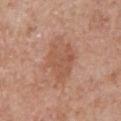biopsy status=total-body-photography surveillance lesion; no biopsy
subject=male, in their 70s
lesion diameter=~5.5 mm (longest diameter)
body site=the chest
image=~15 mm tile from a whole-body skin photo
illumination=white-light illumination